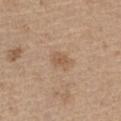Recorded during total-body skin imaging; not selected for excision or biopsy. Captured under white-light illumination. On the left thigh. The lesion-visualizer software estimated an automated nevus-likeness rating near 0 out of 100 and a lesion-detection confidence of about 100/100. This image is a 15 mm lesion crop taken from a total-body photograph. A male subject in their 70s. Measured at roughly 2.5 mm in maximum diameter.